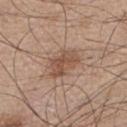Captured during whole-body skin photography for melanoma surveillance; the lesion was not biopsied.
Located on the chest.
Cropped from a whole-body photographic skin survey; the tile spans about 15 mm.
Captured under white-light illumination.
Measured at roughly 4 mm in maximum diameter.
Automated image analysis of the tile measured an outline eccentricity of about 0.8 (0 = round, 1 = elongated) and a shape-asymmetry score of about 0.35 (0 = symmetric). And it measured a lesion color around L≈51 a*≈19 b*≈28 in CIELAB, about 10 CIELAB-L* units darker than the surrounding skin, and a normalized lesion–skin contrast near 7.
The patient is a male roughly 55 years of age.